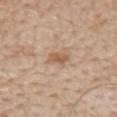No biopsy was performed on this lesion — it was imaged during a full skin examination and was not determined to be concerning. A male subject, aged 58–62. Located on the mid back. A close-up tile cropped from a whole-body skin photograph, about 15 mm across.